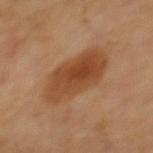Captured during whole-body skin photography for melanoma surveillance; the lesion was not biopsied.
The subject is a male roughly 65 years of age.
A 15 mm close-up tile from a total-body photography series done for melanoma screening.
Located on the upper back.